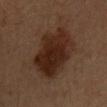An algorithmic analysis of the crop reported a border-irregularity index near 2/10, internal color variation of about 4.5 on a 0–10 scale, and peripheral color asymmetry of about 1.5. The analysis additionally found a classifier nevus-likeness of about 85/100 and a lesion-detection confidence of about 100/100. The recorded lesion diameter is about 7 mm. The subject is a male in their mid- to late 30s. The lesion is located on the chest. A roughly 15 mm field-of-view crop from a total-body skin photograph. Imaged with cross-polarized lighting.